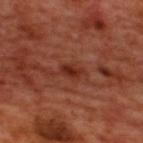Clinical summary: On the upper back. The lesion's longest dimension is about 3 mm. A roughly 15 mm field-of-view crop from a total-body skin photograph. This is a cross-polarized tile. The subject is a male approximately 50 years of age.